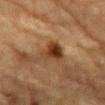Clinical summary: A 15 mm crop from a total-body photograph taken for skin-cancer surveillance. A male subject, aged around 85 or older. The lesion is on the chest. The recorded lesion diameter is about 3.5 mm.